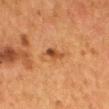{
  "biopsy_status": "not biopsied; imaged during a skin examination",
  "site": "mid back",
  "image": {
    "source": "total-body photography crop",
    "field_of_view_mm": 15
  },
  "patient": {
    "sex": "female",
    "age_approx": 50
  }
}